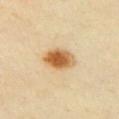Notes:
• workup · total-body-photography surveillance lesion; no biopsy
• lighting · cross-polarized
• image source · ~15 mm crop, total-body skin-cancer survey
• subject · female, roughly 40 years of age
• TBP lesion metrics · an area of roughly 9.5 mm², an eccentricity of roughly 0.7, and a shape-asymmetry score of about 0.15 (0 = symmetric); a nevus-likeness score of about 100/100
• body site · the front of the torso
• lesion diameter · about 4 mm